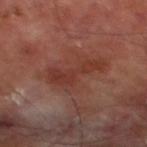{"biopsy_status": "not biopsied; imaged during a skin examination", "image": {"source": "total-body photography crop", "field_of_view_mm": 15}, "site": "left thigh", "patient": {"sex": "male", "age_approx": 70}}